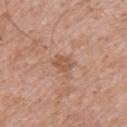Q: Is there a histopathology result?
A: imaged on a skin check; not biopsied
Q: How was this image acquired?
A: 15 mm crop, total-body photography
Q: What are the patient's age and sex?
A: male, about 70 years old
Q: Where on the body is the lesion?
A: the upper back